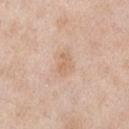Impression:
Imaged during a routine full-body skin examination; the lesion was not biopsied and no histopathology is available.
Clinical summary:
Imaged with white-light lighting. A close-up tile cropped from a whole-body skin photograph, about 15 mm across. A male patient, aged around 55. The lesion is located on the left upper arm.